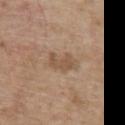| key | value |
|---|---|
| notes | imaged on a skin check; not biopsied |
| subject | male, approximately 70 years of age |
| location | the chest |
| tile lighting | white-light illumination |
| lesion size | about 3.5 mm |
| image | total-body-photography crop, ~15 mm field of view |
| image-analysis metrics | an area of roughly 5.5 mm² and two-axis asymmetry of about 0.35; a nevus-likeness score of about 0/100 |